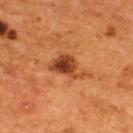image — ~15 mm crop, total-body skin-cancer survey; subject — male, aged 53 to 57; tile lighting — cross-polarized illumination; site — the upper back; lesion size — ≈4.5 mm.